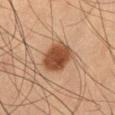Q: Is there a histopathology result?
A: imaged on a skin check; not biopsied
Q: What are the patient's age and sex?
A: male, approximately 50 years of age
Q: What kind of image is this?
A: ~15 mm tile from a whole-body skin photo
Q: What is the anatomic site?
A: the leg
Q: What did automated image analysis measure?
A: an average lesion color of about L≈36 a*≈19 b*≈28 (CIELAB) and a lesion-to-skin contrast of about 11 (normalized; higher = more distinct)
Q: Illumination type?
A: cross-polarized